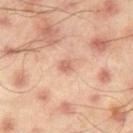notes: no biopsy performed (imaged during a skin exam)
location: the right thigh
acquisition: total-body-photography crop, ~15 mm field of view
image-analysis metrics: an outline eccentricity of about 0.4 (0 = round, 1 = elongated) and two-axis asymmetry of about 0.25; roughly 10 lightness units darker than nearby skin and a normalized border contrast of about 6.5; an automated nevus-likeness rating near 20 out of 100 and a lesion-detection confidence of about 100/100
lighting: cross-polarized illumination
subject: male, aged approximately 45